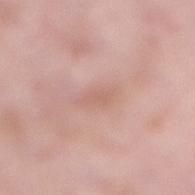notes — total-body-photography surveillance lesion; no biopsy | image — ~15 mm crop, total-body skin-cancer survey | lighting — white-light illumination | diameter — ~3.5 mm (longest diameter) | subject — female, in their 70s | site — the right lower leg.